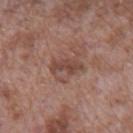| key | value |
|---|---|
| biopsy status | total-body-photography surveillance lesion; no biopsy |
| site | the back |
| acquisition | ~15 mm crop, total-body skin-cancer survey |
| lighting | white-light illumination |
| image-analysis metrics | a lesion area of about 5 mm², a shape eccentricity near 0.9, and a symmetry-axis asymmetry near 0.4; a normalized border contrast of about 7; a border-irregularity rating of about 4.5/10, a within-lesion color-variation index near 2.5/10, and a peripheral color-asymmetry measure near 1; an automated nevus-likeness rating near 0 out of 100 and a lesion-detection confidence of about 100/100 |
| lesion diameter | about 3.5 mm |
| subject | male, aged around 70 |